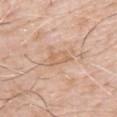biopsy_status: not biopsied; imaged during a skin examination
lighting: white-light
automated_metrics:
  border_irregularity_0_10: 3.5
  peripheral_color_asymmetry: 1.0
  nevus_likeness_0_100: 0
site: chest
patient:
  sex: male
  age_approx: 65
image:
  source: total-body photography crop
  field_of_view_mm: 15
lesion_size:
  long_diameter_mm_approx: 3.0